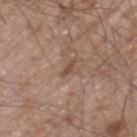A male patient, aged 58 to 62. From the left thigh. This image is a 15 mm lesion crop taken from a total-body photograph.